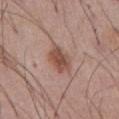No biopsy was performed on this lesion — it was imaged during a full skin examination and was not determined to be concerning.
The lesion is located on the abdomen.
The patient is a male aged 53 to 57.
A 15 mm close-up tile from a total-body photography series done for melanoma screening.